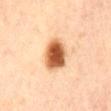Notes:
* imaging modality — ~15 mm crop, total-body skin-cancer survey
* location — the front of the torso
* tile lighting — cross-polarized
* subject — female, aged around 65